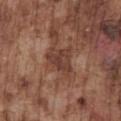Clinical impression: Recorded during total-body skin imaging; not selected for excision or biopsy. Image and clinical context: Cropped from a total-body skin-imaging series; the visible field is about 15 mm. Automated image analysis of the tile measured an average lesion color of about L≈40 a*≈20 b*≈26 (CIELAB), about 9 CIELAB-L* units darker than the surrounding skin, and a normalized lesion–skin contrast near 7.5. From the front of the torso. The recorded lesion diameter is about 3.5 mm. A male patient aged 73 to 77. Captured under white-light illumination.